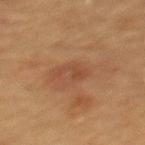Impression: The lesion was photographed on a routine skin check and not biopsied; there is no pathology result. Clinical summary: A close-up tile cropped from a whole-body skin photograph, about 15 mm across. Measured at roughly 4 mm in maximum diameter. Located on the upper back. A female subject aged around 70.